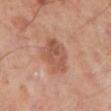{"biopsy_status": "not biopsied; imaged during a skin examination", "automated_metrics": {"cielab_L": 53, "cielab_a": 22, "cielab_b": 30, "vs_skin_darker_L": 9.0, "vs_skin_contrast_norm": 6.5, "border_irregularity_0_10": 2.5, "color_variation_0_10": 4.5}, "site": "left lower leg", "lesion_size": {"long_diameter_mm_approx": 4.5}, "patient": {"sex": "male", "age_approx": 50}, "image": {"source": "total-body photography crop", "field_of_view_mm": 15}, "lighting": "cross-polarized"}